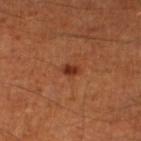The tile uses cross-polarized illumination. A close-up tile cropped from a whole-body skin photograph, about 15 mm across. The lesion is on the left lower leg. The recorded lesion diameter is about 2 mm. An algorithmic analysis of the crop reported a lesion area of about 2 mm², an eccentricity of roughly 0.75, and a shape-asymmetry score of about 0.2 (0 = symmetric). The analysis additionally found a mean CIELAB color near L≈32 a*≈26 b*≈31, roughly 10 lightness units darker than nearby skin, and a lesion-to-skin contrast of about 9.5 (normalized; higher = more distinct). The software also gave a border-irregularity rating of about 2/10. The subject is a male roughly 45 years of age.